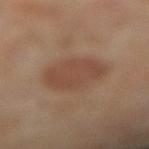| field | value |
|---|---|
| workup | imaged on a skin check; not biopsied |
| illumination | cross-polarized |
| image source | ~15 mm tile from a whole-body skin photo |
| patient | female, aged around 65 |
| location | the left leg |